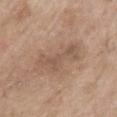Captured during whole-body skin photography for melanoma surveillance; the lesion was not biopsied. On the mid back. Captured under white-light illumination. A female subject, aged 73–77. A lesion tile, about 15 mm wide, cut from a 3D total-body photograph. The lesion's longest dimension is about 6 mm.